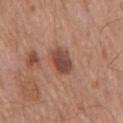The lesion was tiled from a total-body skin photograph and was not biopsied.
Imaged with white-light lighting.
The lesion is on the chest.
A 15 mm close-up extracted from a 3D total-body photography capture.
A male subject roughly 65 years of age.
The lesion-visualizer software estimated a footprint of about 8 mm², a shape eccentricity near 0.7, and a shape-asymmetry score of about 0.15 (0 = symmetric). It also reported border irregularity of about 1.5 on a 0–10 scale and internal color variation of about 4.5 on a 0–10 scale. It also reported a nevus-likeness score of about 60/100 and lesion-presence confidence of about 100/100.
Approximately 3.5 mm at its widest.